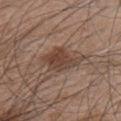<case>
  <biopsy_status>not biopsied; imaged during a skin examination</biopsy_status>
  <automated_metrics>
    <eccentricity>0.65</eccentricity>
    <nevus_likeness_0_100>85</nevus_likeness_0_100>
    <lesion_detection_confidence_0_100>100</lesion_detection_confidence_0_100>
  </automated_metrics>
  <lesion_size>
    <long_diameter_mm_approx>4.5</long_diameter_mm_approx>
  </lesion_size>
  <image>
    <source>total-body photography crop</source>
    <field_of_view_mm>15</field_of_view_mm>
  </image>
  <site>chest</site>
  <lighting>white-light</lighting>
  <patient>
    <sex>male</sex>
    <age_approx>45</age_approx>
  </patient>
</case>